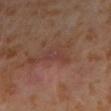site: the left forearm
lesion size: ≈3.5 mm
automated lesion analysis: an area of roughly 4 mm², a shape eccentricity near 0.9, and a symmetry-axis asymmetry near 0.4; a lesion color around L≈37 a*≈21 b*≈23 in CIELAB, roughly 5 lightness units darker than nearby skin, and a normalized lesion–skin contrast near 5
patient: female, aged approximately 50
illumination: cross-polarized illumination
imaging modality: total-body-photography crop, ~15 mm field of view